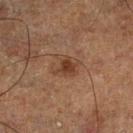This is a cross-polarized tile. This image is a 15 mm lesion crop taken from a total-body photograph. A male subject aged around 65. Longest diameter approximately 3 mm. From the left lower leg.